A roughly 15 mm field-of-view crop from a total-body skin photograph · the lesion is located on the left lower leg · the patient is a male approximately 65 years of age:
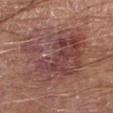diagnosis:
  histopathology: verruca
  malignancy: benign
  taxonomic_path:
    - Benign
    - Inflammatory or infectious diseases
    - Verruca